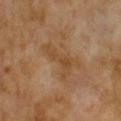A region of skin cropped from a whole-body photographic capture, roughly 15 mm wide. Automated image analysis of the tile measured an outline eccentricity of about 0.9 (0 = round, 1 = elongated). And it measured a classifier nevus-likeness of about 0/100 and lesion-presence confidence of about 100/100. A female patient, aged 58 to 62. This is a cross-polarized tile. From the upper back. The recorded lesion diameter is about 5.5 mm.